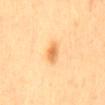Q: Is there a histopathology result?
A: imaged on a skin check; not biopsied
Q: Lesion size?
A: ≈2.5 mm
Q: What kind of image is this?
A: 15 mm crop, total-body photography
Q: Illumination type?
A: cross-polarized
Q: Who is the patient?
A: female, aged 43 to 47
Q: Automated lesion metrics?
A: a lesion color around L≈71 a*≈24 b*≈48 in CIELAB, a lesion–skin lightness drop of about 12, and a lesion-to-skin contrast of about 7.5 (normalized; higher = more distinct); a border-irregularity rating of about 1.5/10, a color-variation rating of about 4/10, and peripheral color asymmetry of about 1.5
Q: Lesion location?
A: the mid back